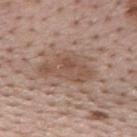Assessment: Recorded during total-body skin imaging; not selected for excision or biopsy. Acquisition and patient details: The subject is a male aged approximately 75. The lesion is on the mid back. A roughly 15 mm field-of-view crop from a total-body skin photograph.